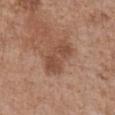{
  "image": {
    "source": "total-body photography crop",
    "field_of_view_mm": 15
  },
  "automated_metrics": {
    "nevus_likeness_0_100": 5,
    "lesion_detection_confidence_0_100": 100
  },
  "site": "chest",
  "lighting": "white-light",
  "patient": {
    "sex": "female",
    "age_approx": 75
  }
}